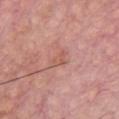Case summary:
• notes · imaged on a skin check; not biopsied
• patient · male, in their mid-60s
• body site · the front of the torso
• diameter · ≈3 mm
• tile lighting · white-light
• automated metrics · a mean CIELAB color near L≈57 a*≈24 b*≈27, a lesion–skin lightness drop of about 6, and a normalized lesion–skin contrast near 4.5; a nevus-likeness score of about 0/100 and a detector confidence of about 100 out of 100 that the crop contains a lesion
• acquisition · ~15 mm tile from a whole-body skin photo